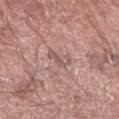{"biopsy_status": "not biopsied; imaged during a skin examination", "automated_metrics": {"area_mm2_approx": 4.0, "eccentricity": 0.85, "shape_asymmetry": 0.45, "cielab_L": 55, "cielab_a": 20, "cielab_b": 22, "vs_skin_darker_L": 8.0, "vs_skin_contrast_norm": 5.5, "nevus_likeness_0_100": 0, "lesion_detection_confidence_0_100": 90}, "patient": {"sex": "male", "age_approx": 75}, "site": "right forearm", "image": {"source": "total-body photography crop", "field_of_view_mm": 15}}